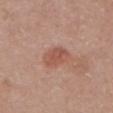The lesion was photographed on a routine skin check and not biopsied; there is no pathology result.
The total-body-photography lesion software estimated a mean CIELAB color near L≈53 a*≈24 b*≈28, about 8 CIELAB-L* units darker than the surrounding skin, and a lesion-to-skin contrast of about 6 (normalized; higher = more distinct). The analysis additionally found an automated nevus-likeness rating near 60 out of 100 and a detector confidence of about 100 out of 100 that the crop contains a lesion.
On the chest.
About 3.5 mm across.
A lesion tile, about 15 mm wide, cut from a 3D total-body photograph.
A female subject roughly 65 years of age.